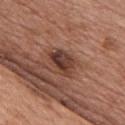A male patient approximately 55 years of age.
A 15 mm close-up extracted from a 3D total-body photography capture.
The lesion is on the chest.